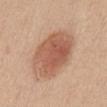Image and clinical context: About 6.5 mm across. A female patient in their 60s. Automated image analysis of the tile measured a footprint of about 27 mm², an eccentricity of roughly 0.6, and a symmetry-axis asymmetry near 0.1. It also reported a lesion color around L≈57 a*≈23 b*≈31 in CIELAB, about 12 CIELAB-L* units darker than the surrounding skin, and a normalized lesion–skin contrast near 8. It also reported lesion-presence confidence of about 100/100. The lesion is located on the abdomen. A 15 mm close-up tile from a total-body photography series done for melanoma screening.